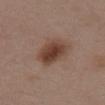| key | value |
|---|---|
| notes | total-body-photography surveillance lesion; no biopsy |
| body site | the front of the torso |
| image source | total-body-photography crop, ~15 mm field of view |
| patient | female, aged 53–57 |
| diameter | ~4 mm (longest diameter) |
| tile lighting | white-light |